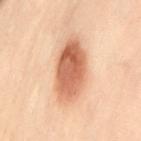Imaged during a routine full-body skin examination; the lesion was not biopsied and no histopathology is available. The total-body-photography lesion software estimated a shape eccentricity near 0.8 and two-axis asymmetry of about 0.15. And it measured an average lesion color of about L≈55 a*≈22 b*≈31 (CIELAB), roughly 14 lightness units darker than nearby skin, and a lesion-to-skin contrast of about 9.5 (normalized; higher = more distinct). The software also gave a border-irregularity rating of about 2/10, internal color variation of about 6 on a 0–10 scale, and peripheral color asymmetry of about 2. From the lower back. Cropped from a whole-body photographic skin survey; the tile spans about 15 mm. A female patient roughly 60 years of age. About 7 mm across.